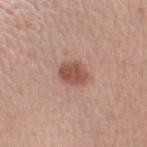notes: catalogued during a skin exam; not biopsied
image source: ~15 mm crop, total-body skin-cancer survey
site: the right upper arm
patient: male, aged around 50